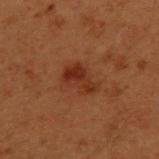| field | value |
|---|---|
| body site | the upper back |
| lighting | cross-polarized |
| patient | male, aged 48–52 |
| size | about 4 mm |
| imaging modality | total-body-photography crop, ~15 mm field of view |